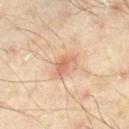<record>
  <biopsy_status>not biopsied; imaged during a skin examination</biopsy_status>
  <automated_metrics>
    <area_mm2_approx>3.5</area_mm2_approx>
    <shape_asymmetry>0.4</shape_asymmetry>
  </automated_metrics>
  <patient>
    <sex>male</sex>
    <age_approx>45</age_approx>
  </patient>
  <site>right lower leg</site>
  <lighting>cross-polarized</lighting>
  <image>
    <source>total-body photography crop</source>
    <field_of_view_mm>15</field_of_view_mm>
  </image>
  <lesion_size>
    <long_diameter_mm_approx>2.5</long_diameter_mm_approx>
  </lesion_size>
</record>